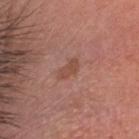Q: Was this lesion biopsied?
A: total-body-photography surveillance lesion; no biopsy
Q: What is the anatomic site?
A: the head or neck
Q: Lesion size?
A: ≈2.5 mm
Q: What lighting was used for the tile?
A: white-light illumination
Q: What are the patient's age and sex?
A: male, roughly 45 years of age
Q: What is the imaging modality?
A: total-body-photography crop, ~15 mm field of view